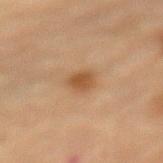Captured during whole-body skin photography for melanoma surveillance; the lesion was not biopsied. Located on the mid back. The subject is a female approximately 80 years of age. A 15 mm crop from a total-body photograph taken for skin-cancer surveillance. Imaged with cross-polarized lighting. Longest diameter approximately 2.5 mm.